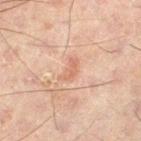Clinical impression:
The lesion was tiled from a total-body skin photograph and was not biopsied.
Clinical summary:
A male patient aged around 60. The tile uses cross-polarized illumination. A close-up tile cropped from a whole-body skin photograph, about 15 mm across. On the left thigh. The total-body-photography lesion software estimated a lesion area of about 4 mm², a shape eccentricity near 0.85, and two-axis asymmetry of about 0.45. The analysis additionally found a border-irregularity index near 4.5/10, a color-variation rating of about 1/10, and a peripheral color-asymmetry measure near 0. The software also gave a classifier nevus-likeness of about 0/100 and a lesion-detection confidence of about 100/100.